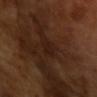Image and clinical context: Cropped from a whole-body photographic skin survey; the tile spans about 15 mm. Imaged with cross-polarized lighting. The recorded lesion diameter is about 3 mm. A male patient, in their mid- to late 60s.